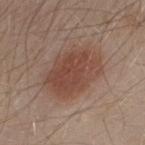No biopsy was performed on this lesion — it was imaged during a full skin examination and was not determined to be concerning. This is a white-light tile. The lesion is on the upper back. The subject is a male in their 30s. A region of skin cropped from a whole-body photographic capture, roughly 15 mm wide.